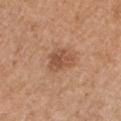Findings:
– biopsy status: no biopsy performed (imaged during a skin exam)
– subject: female, aged 38–42
– imaging modality: total-body-photography crop, ~15 mm field of view
– anatomic site: the chest
– lighting: white-light
– diameter: ~3.5 mm (longest diameter)